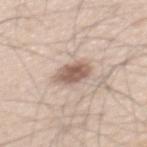Acquisition and patient details: A region of skin cropped from a whole-body photographic capture, roughly 15 mm wide. A male subject aged 58–62. Automated tile analysis of the lesion measured an eccentricity of roughly 0.7 and a symmetry-axis asymmetry near 0.15. It also reported about 14 CIELAB-L* units darker than the surrounding skin and a lesion-to-skin contrast of about 9 (normalized; higher = more distinct). Located on the mid back. Measured at roughly 3.5 mm in maximum diameter. Imaged with white-light lighting.